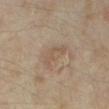biopsy status: imaged on a skin check; not biopsied | body site: the right lower leg | subject: female, in their mid- to late 30s | imaging modality: ~15 mm tile from a whole-body skin photo | automated metrics: about 6 CIELAB-L* units darker than the surrounding skin; a border-irregularity rating of about 7/10, a color-variation rating of about 0/10, and radial color variation of about 0; a classifier nevus-likeness of about 0/100 and lesion-presence confidence of about 100/100 | size: about 4 mm.